A female patient, roughly 40 years of age; cropped from a whole-body photographic skin survey; the tile spans about 15 mm; from the back: 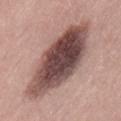| key | value |
|---|---|
| tile lighting | white-light illumination |
| lesion diameter | about 11.5 mm |
| TBP lesion metrics | an area of roughly 41 mm² and an eccentricity of roughly 0.9; a lesion color around L≈47 a*≈19 b*≈20 in CIELAB, about 20 CIELAB-L* units darker than the surrounding skin, and a lesion-to-skin contrast of about 13.5 (normalized; higher = more distinct); an automated nevus-likeness rating near 40 out of 100 and a detector confidence of about 100 out of 100 that the crop contains a lesion |
| diagnosis | a dysplastic (Clark) nevus |